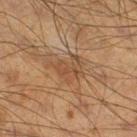{
  "biopsy_status": "not biopsied; imaged during a skin examination",
  "patient": {
    "sex": "male",
    "age_approx": 60
  },
  "lesion_size": {
    "long_diameter_mm_approx": 4.0
  },
  "site": "right thigh",
  "image": {
    "source": "total-body photography crop",
    "field_of_view_mm": 15
  },
  "lighting": "cross-polarized"
}